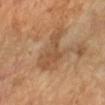No biopsy was performed on this lesion — it was imaged during a full skin examination and was not determined to be concerning.
Measured at roughly 5.5 mm in maximum diameter.
The subject is a female aged 68–72.
Automated tile analysis of the lesion measured an outline eccentricity of about 0.75 (0 = round, 1 = elongated) and two-axis asymmetry of about 0.55. The analysis additionally found lesion-presence confidence of about 100/100.
Located on the left forearm.
A 15 mm close-up extracted from a 3D total-body photography capture.